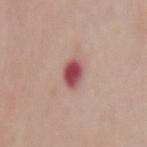The lesion was tiled from a total-body skin photograph and was not biopsied. This is a white-light tile. Longest diameter approximately 2.5 mm. An algorithmic analysis of the crop reported an average lesion color of about L≈50 a*≈30 b*≈21 (CIELAB), a lesion–skin lightness drop of about 16, and a lesion-to-skin contrast of about 11.5 (normalized; higher = more distinct). The analysis additionally found border irregularity of about 1.5 on a 0–10 scale and a color-variation rating of about 5/10. The analysis additionally found a lesion-detection confidence of about 100/100. A male patient roughly 55 years of age. The lesion is located on the mid back. A close-up tile cropped from a whole-body skin photograph, about 15 mm across.